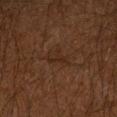Imaged during a routine full-body skin examination; the lesion was not biopsied and no histopathology is available. From the left forearm. A lesion tile, about 15 mm wide, cut from a 3D total-body photograph. About 3.5 mm across. A male patient in their mid-60s. Automated tile analysis of the lesion measured an area of roughly 4 mm², a shape eccentricity near 0.85, and two-axis asymmetry of about 0.5. The analysis additionally found a nevus-likeness score of about 5/100 and a lesion-detection confidence of about 85/100.